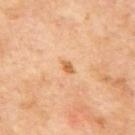Imaged during a routine full-body skin examination; the lesion was not biopsied and no histopathology is available. The lesion is on the back. The tile uses cross-polarized illumination. The lesion's longest dimension is about 2 mm. The total-body-photography lesion software estimated a border-irregularity rating of about 3/10, internal color variation of about 0 on a 0–10 scale, and radial color variation of about 0. A male patient, aged around 70. Cropped from a whole-body photographic skin survey; the tile spans about 15 mm.